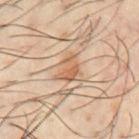workup: imaged on a skin check; not biopsied | subject: male, approximately 40 years of age | location: the chest | illumination: cross-polarized | TBP lesion metrics: an average lesion color of about L≈59 a*≈21 b*≈34 (CIELAB), about 10 CIELAB-L* units darker than the surrounding skin, and a lesion-to-skin contrast of about 7.5 (normalized; higher = more distinct) | image: ~15 mm tile from a whole-body skin photo.